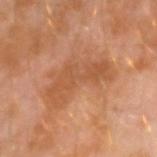No biopsy was performed on this lesion — it was imaged during a full skin examination and was not determined to be concerning. Measured at roughly 6.5 mm in maximum diameter. The total-body-photography lesion software estimated a footprint of about 12 mm², a shape eccentricity near 0.95, and a symmetry-axis asymmetry near 0.6. The software also gave a lesion-detection confidence of about 100/100. From the left arm. A lesion tile, about 15 mm wide, cut from a 3D total-body photograph. A male subject approximately 30 years of age.